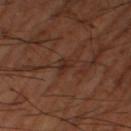Acquisition and patient details:
The tile uses cross-polarized illumination. Cropped from a whole-body photographic skin survey; the tile spans about 15 mm. A male subject in their mid- to late 60s. The recorded lesion diameter is about 2.5 mm. An algorithmic analysis of the crop reported a lesion area of about 3 mm², an eccentricity of roughly 0.8, and a shape-asymmetry score of about 0.4 (0 = symmetric). The software also gave a lesion-to-skin contrast of about 6.5 (normalized; higher = more distinct). The software also gave a border-irregularity rating of about 3.5/10 and peripheral color asymmetry of about 0.5. Located on the right thigh.